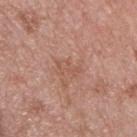Impression: No biopsy was performed on this lesion — it was imaged during a full skin examination and was not determined to be concerning. Background: Imaged with white-light lighting. From the chest. This image is a 15 mm lesion crop taken from a total-body photograph. The subject is a male about 50 years old. Measured at roughly 3 mm in maximum diameter.